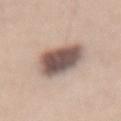biopsy status: no biopsy performed (imaged during a skin exam) | tile lighting: white-light | patient: female, aged approximately 45 | automated lesion analysis: a footprint of about 17 mm², a shape eccentricity near 0.65, and a shape-asymmetry score of about 0.2 (0 = symmetric); roughly 19 lightness units darker than nearby skin; an automated nevus-likeness rating near 40 out of 100 and a detector confidence of about 100 out of 100 that the crop contains a lesion | site: the lower back | lesion size: ~5 mm (longest diameter) | image source: ~15 mm crop, total-body skin-cancer survey.